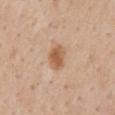Q: Was this lesion biopsied?
A: total-body-photography surveillance lesion; no biopsy
Q: What lighting was used for the tile?
A: white-light illumination
Q: Lesion size?
A: ≈3 mm
Q: Lesion location?
A: the mid back
Q: Automated lesion metrics?
A: an average lesion color of about L≈57 a*≈21 b*≈34 (CIELAB), about 12 CIELAB-L* units darker than the surrounding skin, and a normalized lesion–skin contrast near 8.5
Q: Who is the patient?
A: male, aged 53–57
Q: What is the imaging modality?
A: total-body-photography crop, ~15 mm field of view